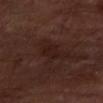<lesion>
<lesion_size>
  <long_diameter_mm_approx>3.0</long_diameter_mm_approx>
</lesion_size>
<automated_metrics>
  <area_mm2_approx>4.0</area_mm2_approx>
  <eccentricity>0.85</eccentricity>
  <shape_asymmetry>0.3</shape_asymmetry>
  <border_irregularity_0_10>3.0</border_irregularity_0_10>
  <color_variation_0_10>1.5</color_variation_0_10>
  <nevus_likeness_0_100>0</nevus_likeness_0_100>
</automated_metrics>
<image>
  <source>total-body photography crop</source>
  <field_of_view_mm>15</field_of_view_mm>
</image>
<site>left forearm</site>
<lighting>cross-polarized</lighting>
</lesion>